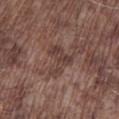biopsy status = imaged on a skin check; not biopsied | location = the left lower leg | diameter = ≈4 mm | subject = male, in their mid-70s | lighting = white-light | acquisition = ~15 mm crop, total-body skin-cancer survey.